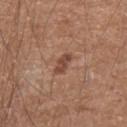Captured during whole-body skin photography for melanoma surveillance; the lesion was not biopsied. The lesion is located on the right forearm. A 15 mm crop from a total-body photograph taken for skin-cancer surveillance. The total-body-photography lesion software estimated a mean CIELAB color near L≈46 a*≈21 b*≈28, roughly 10 lightness units darker than nearby skin, and a normalized border contrast of about 7.5. The analysis additionally found border irregularity of about 3 on a 0–10 scale, a within-lesion color-variation index near 1.5/10, and radial color variation of about 0.5. And it measured a detector confidence of about 100 out of 100 that the crop contains a lesion. A male subject, aged 53–57. Longest diameter approximately 3 mm.